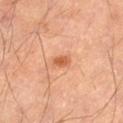biopsy_status: not biopsied; imaged during a skin examination
image:
  source: total-body photography crop
  field_of_view_mm: 15
patient:
  sex: male
  age_approx: 55
site: left thigh